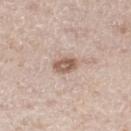Impression: Captured during whole-body skin photography for melanoma surveillance; the lesion was not biopsied. Context: Approximately 2.5 mm at its widest. A region of skin cropped from a whole-body photographic capture, roughly 15 mm wide. The lesion is on the right thigh. Imaged with white-light lighting. A male subject approximately 65 years of age. Automated image analysis of the tile measured a footprint of about 4.5 mm², an outline eccentricity of about 0.75 (0 = round, 1 = elongated), and two-axis asymmetry of about 0.2. It also reported a mean CIELAB color near L≈58 a*≈18 b*≈26, roughly 13 lightness units darker than nearby skin, and a lesion-to-skin contrast of about 9 (normalized; higher = more distinct). The analysis additionally found border irregularity of about 1.5 on a 0–10 scale and peripheral color asymmetry of about 1.5.